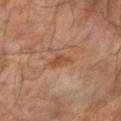Q: Is there a histopathology result?
A: no biopsy performed (imaged during a skin exam)
Q: What kind of image is this?
A: ~15 mm crop, total-body skin-cancer survey
Q: Lesion size?
A: ≈2.5 mm
Q: What did automated image analysis measure?
A: lesion-presence confidence of about 100/100
Q: Lesion location?
A: the right forearm
Q: Who is the patient?
A: male, aged 63–67